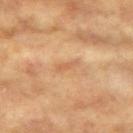– biopsy status: total-body-photography surveillance lesion; no biopsy
– site: the left upper arm
– patient: female, about 75 years old
– imaging modality: 15 mm crop, total-body photography
– illumination: cross-polarized illumination
– diameter: ~2.5 mm (longest diameter)